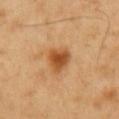Case summary:
* notes — catalogued during a skin exam; not biopsied
* acquisition — ~15 mm crop, total-body skin-cancer survey
* lesion size — about 3.5 mm
* illumination — cross-polarized illumination
* site — the front of the torso
* subject — male, about 50 years old